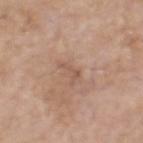{
  "biopsy_status": "not biopsied; imaged during a skin examination",
  "lighting": "white-light",
  "site": "head or neck",
  "lesion_size": {
    "long_diameter_mm_approx": 3.0
  },
  "patient": {
    "sex": "male",
    "age_approx": 70
  },
  "image": {
    "source": "total-body photography crop",
    "field_of_view_mm": 15
  }
}